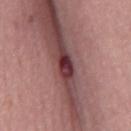Findings:
- notes — catalogued during a skin exam; not biopsied
- size — ~2.5 mm (longest diameter)
- subject — male, in their mid-70s
- lighting — white-light
- image source — ~15 mm crop, total-body skin-cancer survey
- body site — the mid back